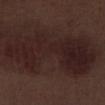{
  "biopsy_status": "not biopsied; imaged during a skin examination",
  "site": "left lower leg",
  "automated_metrics": {
    "area_mm2_approx": 50.0,
    "eccentricity": 0.9,
    "shape_asymmetry": 0.3,
    "cielab_L": 20,
    "cielab_a": 16,
    "cielab_b": 15,
    "vs_skin_contrast_norm": 8.5,
    "nevus_likeness_0_100": 0,
    "lesion_detection_confidence_0_100": 100
  },
  "lighting": "white-light",
  "image": {
    "source": "total-body photography crop",
    "field_of_view_mm": 15
  },
  "lesion_size": {
    "long_diameter_mm_approx": 12.5
  },
  "patient": {
    "sex": "male",
    "age_approx": 70
  }
}